A close-up tile cropped from a whole-body skin photograph, about 15 mm across. Captured under white-light illumination. A male subject, in their mid-40s. Located on the right upper arm: 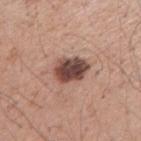{"diagnosis": {"histopathology": "dysplastic (Clark) nevus", "malignancy": "benign", "taxonomic_path": ["Benign", "Benign melanocytic proliferations", "Nevus", "Nevus, Atypical, Dysplastic, or Clark"]}}